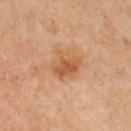Q: Is there a histopathology result?
A: no biopsy performed (imaged during a skin exam)
Q: What kind of image is this?
A: ~15 mm crop, total-body skin-cancer survey
Q: Where on the body is the lesion?
A: the front of the torso
Q: Lesion size?
A: ~3.5 mm (longest diameter)
Q: Patient demographics?
A: female, approximately 40 years of age